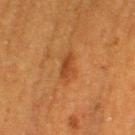<case>
<biopsy_status>not biopsied; imaged during a skin examination</biopsy_status>
<image>
  <source>total-body photography crop</source>
  <field_of_view_mm>15</field_of_view_mm>
</image>
<lesion_size>
  <long_diameter_mm_approx>3.5</long_diameter_mm_approx>
</lesion_size>
<patient>
  <sex>female</sex>
  <age_approx>40</age_approx>
</patient>
<lighting>cross-polarized</lighting>
<automated_metrics>
  <area_mm2_approx>5.5</area_mm2_approx>
  <eccentricity>0.85</eccentricity>
  <shape_asymmetry>0.25</shape_asymmetry>
</automated_metrics>
<site>left thigh</site>
</case>